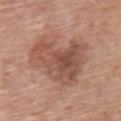tile lighting: white-light illumination
subject: male, about 60 years old
automated lesion analysis: a lesion area of about 29 mm²; a mean CIELAB color near L≈52 a*≈22 b*≈27, about 10 CIELAB-L* units darker than the surrounding skin, and a normalized border contrast of about 7; a border-irregularity index near 5/10 and a within-lesion color-variation index near 5.5/10
anatomic site: the upper back
size: ≈7 mm
image: ~15 mm tile from a whole-body skin photo